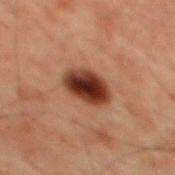Image and clinical context:
Captured under cross-polarized illumination. A 15 mm close-up extracted from a 3D total-body photography capture. A male subject, aged around 60. From the mid back. Longest diameter approximately 4 mm.